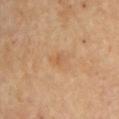{"site": "right upper arm", "lighting": "cross-polarized", "image": {"source": "total-body photography crop", "field_of_view_mm": 15}, "patient": {"sex": "female", "age_approx": 65}, "lesion_size": {"long_diameter_mm_approx": 2.5}}